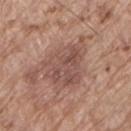notes — total-body-photography surveillance lesion; no biopsy | body site — the lower back | subject — male, about 80 years old | image — total-body-photography crop, ~15 mm field of view.